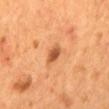Findings:
- biopsy status: imaged on a skin check; not biopsied
- acquisition: 15 mm crop, total-body photography
- subject: male, aged 53–57
- lighting: cross-polarized illumination
- body site: the back
- size: about 2.5 mm
- automated metrics: a mean CIELAB color near L≈50 a*≈27 b*≈38, a lesion–skin lightness drop of about 13, and a lesion-to-skin contrast of about 9 (normalized; higher = more distinct); a border-irregularity rating of about 2.5/10 and internal color variation of about 2 on a 0–10 scale; a nevus-likeness score of about 90/100 and lesion-presence confidence of about 100/100